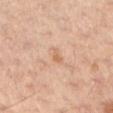anatomic site — the arm
acquisition — ~15 mm crop, total-body skin-cancer survey
subject — female, aged around 55